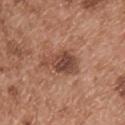Q: Was a biopsy performed?
A: total-body-photography surveillance lesion; no biopsy
Q: Lesion size?
A: about 5 mm
Q: How was the tile lit?
A: white-light
Q: How was this image acquired?
A: ~15 mm tile from a whole-body skin photo
Q: What are the patient's age and sex?
A: male, aged 53–57
Q: What is the anatomic site?
A: the upper back